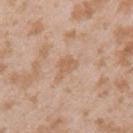Clinical impression: No biopsy was performed on this lesion — it was imaged during a full skin examination and was not determined to be concerning. Background: A 15 mm close-up extracted from a 3D total-body photography capture. Automated tile analysis of the lesion measured an outline eccentricity of about 0.8 (0 = round, 1 = elongated) and a shape-asymmetry score of about 0.5 (0 = symmetric). The software also gave a lesion-detection confidence of about 100/100. Captured under white-light illumination. The subject is a female about 25 years old. The lesion is located on the left upper arm.